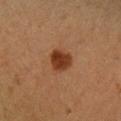biopsy status: total-body-photography surveillance lesion; no biopsy | patient: female, in their mid- to late 40s | illumination: cross-polarized illumination | image source: ~15 mm crop, total-body skin-cancer survey | site: the right forearm | image-analysis metrics: an area of roughly 6.5 mm², a shape eccentricity near 0.45, and a shape-asymmetry score of about 0.25 (0 = symmetric); an average lesion color of about L≈32 a*≈22 b*≈30 (CIELAB), about 11 CIELAB-L* units darker than the surrounding skin, and a normalized lesion–skin contrast near 10.5 | diameter: about 3 mm.